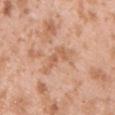Imaged during a routine full-body skin examination; the lesion was not biopsied and no histopathology is available. The recorded lesion diameter is about 4.5 mm. A 15 mm crop from a total-body photograph taken for skin-cancer surveillance. This is a white-light tile. The patient is a male aged 23 to 27. The total-body-photography lesion software estimated a mean CIELAB color near L≈61 a*≈22 b*≈34, about 8 CIELAB-L* units darker than the surrounding skin, and a lesion-to-skin contrast of about 5.5 (normalized; higher = more distinct). Located on the right upper arm.